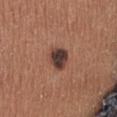Part of a total-body skin-imaging series; this lesion was reviewed on a skin check and was not flagged for biopsy. From the abdomen. A lesion tile, about 15 mm wide, cut from a 3D total-body photograph. The lesion-visualizer software estimated a footprint of about 6 mm², an eccentricity of roughly 0.55, and a symmetry-axis asymmetry near 0.2. The software also gave roughly 16 lightness units darker than nearby skin and a normalized lesion–skin contrast near 13.5. Longest diameter approximately 3 mm. The tile uses white-light illumination. A female subject approximately 35 years of age.